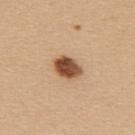Q: Was a biopsy performed?
A: total-body-photography surveillance lesion; no biopsy
Q: Where on the body is the lesion?
A: the upper back
Q: What kind of image is this?
A: 15 mm crop, total-body photography
Q: Patient demographics?
A: female, aged 43 to 47